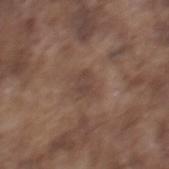Notes:
• biopsy status: imaged on a skin check; not biopsied
• acquisition: ~15 mm tile from a whole-body skin photo
• TBP lesion metrics: a border-irregularity rating of about 3/10; a nevus-likeness score of about 0/100 and a lesion-detection confidence of about 100/100
• patient: male, aged around 75
• location: the mid back
• illumination: white-light illumination
• diameter: ≈2.5 mm